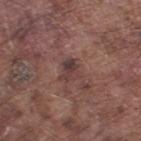Assessment:
The lesion was tiled from a total-body skin photograph and was not biopsied.
Clinical summary:
The lesion is on the right thigh. Automated image analysis of the tile measured a border-irregularity index near 3.5/10, a within-lesion color-variation index near 6/10, and radial color variation of about 2. It also reported a nevus-likeness score of about 0/100 and lesion-presence confidence of about 100/100. A male subject, in their mid- to late 70s. Captured under white-light illumination. Cropped from a total-body skin-imaging series; the visible field is about 15 mm. Longest diameter approximately 3 mm.